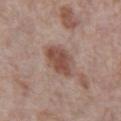No biopsy was performed on this lesion — it was imaged during a full skin examination and was not determined to be concerning. The lesion is on the abdomen. A 15 mm crop from a total-body photograph taken for skin-cancer surveillance. The lesion's longest dimension is about 4.5 mm. A male subject in their 70s. This is a white-light tile.